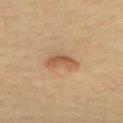No biopsy was performed on this lesion — it was imaged during a full skin examination and was not determined to be concerning. A female patient, aged around 40. This image is a 15 mm lesion crop taken from a total-body photograph. From the mid back. Longest diameter approximately 4 mm. Automated image analysis of the tile measured a lesion area of about 5.5 mm² and a symmetry-axis asymmetry near 0.4. It also reported a lesion color around L≈46 a*≈18 b*≈30 in CIELAB and roughly 9 lightness units darker than nearby skin. The software also gave border irregularity of about 4.5 on a 0–10 scale and radial color variation of about 1. Captured under cross-polarized illumination.